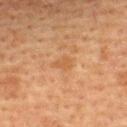Part of a total-body skin-imaging series; this lesion was reviewed on a skin check and was not flagged for biopsy. The recorded lesion diameter is about 2.5 mm. The lesion is located on the upper back. The subject is a female in their mid- to late 50s. A lesion tile, about 15 mm wide, cut from a 3D total-body photograph.